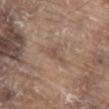Impression:
The lesion was photographed on a routine skin check and not biopsied; there is no pathology result.
Context:
The lesion's longest dimension is about 2.5 mm. Cropped from a total-body skin-imaging series; the visible field is about 15 mm. A male patient aged 78 to 82. The lesion is located on the abdomen.